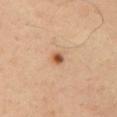Impression: The lesion was photographed on a routine skin check and not biopsied; there is no pathology result. Context: Approximately 1.5 mm at its widest. The patient is a male aged 63–67. A lesion tile, about 15 mm wide, cut from a 3D total-body photograph. From the back. This is a cross-polarized tile.